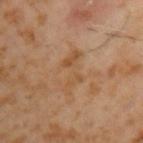Assessment: The lesion was photographed on a routine skin check and not biopsied; there is no pathology result. Background: A male patient, aged 58 to 62. A region of skin cropped from a whole-body photographic capture, roughly 15 mm wide. From the left upper arm.